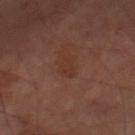Q: Is there a histopathology result?
A: no biopsy performed (imaged during a skin exam)
Q: What is the lesion's diameter?
A: ≈2.5 mm
Q: What are the patient's age and sex?
A: male, aged around 70
Q: What lighting was used for the tile?
A: cross-polarized illumination
Q: How was this image acquired?
A: ~15 mm tile from a whole-body skin photo
Q: What is the anatomic site?
A: the right lower leg